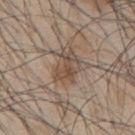{"biopsy_status": "not biopsied; imaged during a skin examination", "image": {"source": "total-body photography crop", "field_of_view_mm": 15}, "lighting": "white-light", "lesion_size": {"long_diameter_mm_approx": 3.5}, "site": "mid back", "patient": {"sex": "male", "age_approx": 45}}